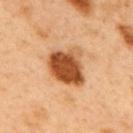Clinical impression: No biopsy was performed on this lesion — it was imaged during a full skin examination and was not determined to be concerning. Image and clinical context: Captured under cross-polarized illumination. A 15 mm crop from a total-body photograph taken for skin-cancer surveillance. Measured at roughly 4.5 mm in maximum diameter. The patient is a male aged 48–52. On the upper back.